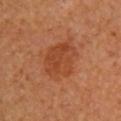Clinical impression: Recorded during total-body skin imaging; not selected for excision or biopsy. Acquisition and patient details: On the upper back. A 15 mm crop from a total-body photograph taken for skin-cancer surveillance. The lesion's longest dimension is about 4.5 mm. The subject is female.